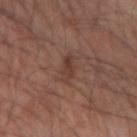No biopsy was performed on this lesion — it was imaged during a full skin examination and was not determined to be concerning. A male subject about 65 years old. About 3 mm across. On the right forearm. This image is a 15 mm lesion crop taken from a total-body photograph. The tile uses cross-polarized illumination.